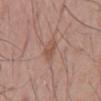Q: Was this lesion biopsied?
A: imaged on a skin check; not biopsied
Q: How large is the lesion?
A: about 3 mm
Q: What did automated image analysis measure?
A: an area of roughly 4.5 mm² and an eccentricity of roughly 0.7; a lesion color around L≈52 a*≈20 b*≈27 in CIELAB; a border-irregularity rating of about 2.5/10, internal color variation of about 2 on a 0–10 scale, and radial color variation of about 1
Q: What kind of image is this?
A: 15 mm crop, total-body photography
Q: Where on the body is the lesion?
A: the back
Q: Who is the patient?
A: male, in their mid-50s
Q: What lighting was used for the tile?
A: white-light